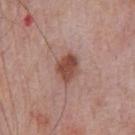<case>
  <biopsy_status>not biopsied; imaged during a skin examination</biopsy_status>
  <patient>
    <sex>male</sex>
    <age_approx>75</age_approx>
  </patient>
  <lesion_size>
    <long_diameter_mm_approx>3.5</long_diameter_mm_approx>
  </lesion_size>
  <image>
    <source>total-body photography crop</source>
    <field_of_view_mm>15</field_of_view_mm>
  </image>
  <site>chest</site>
</case>